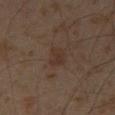Assessment: The lesion was tiled from a total-body skin photograph and was not biopsied. Clinical summary: A male patient aged 58 to 62. This image is a 15 mm lesion crop taken from a total-body photograph. Longest diameter approximately 3 mm. The lesion is located on the leg. Imaged with cross-polarized lighting.